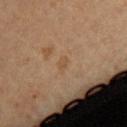Q: Was this lesion biopsied?
A: catalogued during a skin exam; not biopsied
Q: Patient demographics?
A: female, aged 28 to 32
Q: Where on the body is the lesion?
A: the back
Q: How was this image acquired?
A: total-body-photography crop, ~15 mm field of view
Q: How was the tile lit?
A: cross-polarized